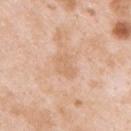Case summary:
– biopsy status: total-body-photography surveillance lesion; no biopsy